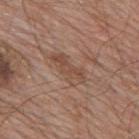Recorded during total-body skin imaging; not selected for excision or biopsy.
The lesion-visualizer software estimated a classifier nevus-likeness of about 0/100 and a detector confidence of about 100 out of 100 that the crop contains a lesion.
From the back.
Cropped from a total-body skin-imaging series; the visible field is about 15 mm.
Measured at roughly 5 mm in maximum diameter.
This is a white-light tile.
The subject is a male aged 78 to 82.